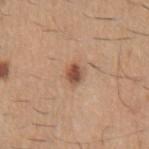Q: Was a biopsy performed?
A: catalogued during a skin exam; not biopsied
Q: Who is the patient?
A: male, in their 60s
Q: Lesion size?
A: ≈2.5 mm
Q: What kind of image is this?
A: total-body-photography crop, ~15 mm field of view
Q: What is the anatomic site?
A: the left upper arm